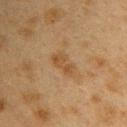{"biopsy_status": "not biopsied; imaged during a skin examination", "patient": {"sex": "female", "age_approx": 40}, "lesion_size": {"long_diameter_mm_approx": 3.5}, "lighting": "cross-polarized", "site": "upper back", "image": {"source": "total-body photography crop", "field_of_view_mm": 15}, "automated_metrics": {"area_mm2_approx": 5.0, "eccentricity": 0.85, "vs_skin_darker_L": 6.0, "vs_skin_contrast_norm": 6.0}}